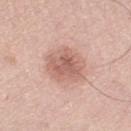| key | value |
|---|---|
| workup | total-body-photography surveillance lesion; no biopsy |
| patient | male, in their mid-60s |
| acquisition | ~15 mm tile from a whole-body skin photo |
| anatomic site | the right thigh |